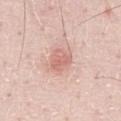biopsy_status: not biopsied; imaged during a skin examination
image:
  source: total-body photography crop
  field_of_view_mm: 15
automated_metrics:
  cielab_L: 66
  cielab_a: 24
  cielab_b: 26
  vs_skin_darker_L: 9.0
  border_irregularity_0_10: 2.0
  peripheral_color_asymmetry: 1.0
lesion_size:
  long_diameter_mm_approx: 3.0
site: chest
patient:
  sex: male
  age_approx: 50